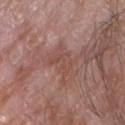{"biopsy_status": "not biopsied; imaged during a skin examination"}